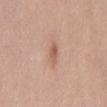Recorded during total-body skin imaging; not selected for excision or biopsy.
The tile uses white-light illumination.
The lesion is on the abdomen.
A region of skin cropped from a whole-body photographic capture, roughly 15 mm wide.
Automated image analysis of the tile measured a lesion area of about 3 mm², an outline eccentricity of about 0.85 (0 = round, 1 = elongated), and a shape-asymmetry score of about 0.3 (0 = symmetric). The analysis additionally found a lesion color around L≈58 a*≈23 b*≈29 in CIELAB and a normalized lesion–skin contrast near 6.5. It also reported a border-irregularity index near 3/10 and radial color variation of about 1. And it measured a classifier nevus-likeness of about 40/100 and a detector confidence of about 100 out of 100 that the crop contains a lesion.
Measured at roughly 2.5 mm in maximum diameter.
The patient is a female in their mid- to late 30s.